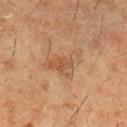Findings:
* follow-up · total-body-photography surveillance lesion; no biopsy
* site · the right lower leg
* subject · female, aged around 50
* tile lighting · cross-polarized illumination
* automated lesion analysis · a border-irregularity rating of about 6/10, a within-lesion color-variation index near 4.5/10, and radial color variation of about 1.5; an automated nevus-likeness rating near 0 out of 100
* image · 15 mm crop, total-body photography